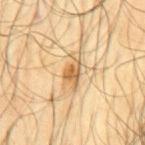acquisition: ~15 mm tile from a whole-body skin photo; patient: male, roughly 65 years of age; location: the mid back; lesion size: ~4.5 mm (longest diameter); lighting: cross-polarized illumination.